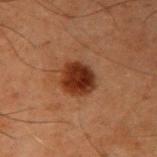acquisition — ~15 mm crop, total-body skin-cancer survey | subject — male, aged approximately 60 | illumination — cross-polarized illumination | anatomic site — the arm | image-analysis metrics — an area of roughly 10 mm², an outline eccentricity of about 0.45 (0 = round, 1 = elongated), and two-axis asymmetry of about 0.1; a lesion color around L≈25 a*≈21 b*≈26 in CIELAB and about 12 CIELAB-L* units darker than the surrounding skin; a border-irregularity index near 1/10, a color-variation rating of about 4.5/10, and peripheral color asymmetry of about 1.5; an automated nevus-likeness rating near 100 out of 100 and lesion-presence confidence of about 100/100 | diameter — ~4 mm (longest diameter).